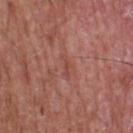- notes — catalogued during a skin exam; not biopsied